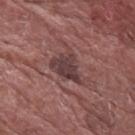This lesion was catalogued during total-body skin photography and was not selected for biopsy.
Imaged with white-light lighting.
The patient is a male about 75 years old.
Cropped from a whole-body photographic skin survey; the tile spans about 15 mm.
The lesion is on the left forearm.
Longest diameter approximately 4.5 mm.